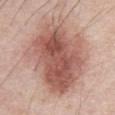– workup — catalogued during a skin exam; not biopsied
– patient — male, aged 68 to 72
– TBP lesion metrics — an automated nevus-likeness rating near 70 out of 100 and a lesion-detection confidence of about 100/100
– acquisition — ~15 mm tile from a whole-body skin photo
– body site — the abdomen
– lighting — white-light
– lesion size — ~9.5 mm (longest diameter)